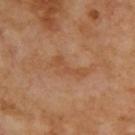No biopsy was performed on this lesion — it was imaged during a full skin examination and was not determined to be concerning. On the back. Automated image analysis of the tile measured an area of roughly 5 mm², an eccentricity of roughly 0.95, and a shape-asymmetry score of about 0.6 (0 = symmetric). The analysis additionally found roughly 6 lightness units darker than nearby skin and a lesion-to-skin contrast of about 5 (normalized; higher = more distinct). The analysis additionally found a nevus-likeness score of about 0/100 and lesion-presence confidence of about 100/100. A male patient approximately 70 years of age. Measured at roughly 4.5 mm in maximum diameter. Captured under cross-polarized illumination. A lesion tile, about 15 mm wide, cut from a 3D total-body photograph.